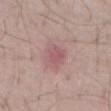{"biopsy_status": "not biopsied; imaged during a skin examination", "image": {"source": "total-body photography crop", "field_of_view_mm": 15}, "site": "chest", "patient": {"sex": "male", "age_approx": 65}, "lighting": "white-light", "lesion_size": {"long_diameter_mm_approx": 2.5}, "automated_metrics": {"area_mm2_approx": 5.0, "eccentricity": 0.4, "shape_asymmetry": 0.2, "cielab_L": 55, "cielab_a": 24, "cielab_b": 18, "vs_skin_darker_L": 7.0, "vs_skin_contrast_norm": 5.0, "border_irregularity_0_10": 1.5, "nevus_likeness_0_100": 0, "lesion_detection_confidence_0_100": 100}}